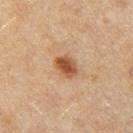Notes:
- workup — total-body-photography surveillance lesion; no biopsy
- patient — female, about 60 years old
- acquisition — total-body-photography crop, ~15 mm field of view
- image-analysis metrics — an area of roughly 5 mm², a shape eccentricity near 0.75, and a shape-asymmetry score of about 0.2 (0 = symmetric); a border-irregularity rating of about 2/10, a within-lesion color-variation index near 3.5/10, and peripheral color asymmetry of about 1; lesion-presence confidence of about 100/100
- location — the right thigh
- lesion diameter — ~3 mm (longest diameter)
- lighting — cross-polarized illumination